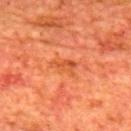Clinical impression:
Captured during whole-body skin photography for melanoma surveillance; the lesion was not biopsied.
Clinical summary:
The subject is a male in their mid-60s. From the upper back. Measured at roughly 3.5 mm in maximum diameter. Captured under cross-polarized illumination. A lesion tile, about 15 mm wide, cut from a 3D total-body photograph. Automated tile analysis of the lesion measured an eccentricity of roughly 0.9 and two-axis asymmetry of about 0.35. It also reported an average lesion color of about L≈50 a*≈33 b*≈42 (CIELAB) and a lesion–skin lightness drop of about 7. And it measured a lesion-detection confidence of about 100/100.